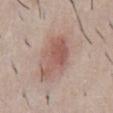Imaged during a routine full-body skin examination; the lesion was not biopsied and no histopathology is available.
Automated image analysis of the tile measured a footprint of about 13 mm². It also reported a border-irregularity index near 4/10 and internal color variation of about 4 on a 0–10 scale.
A 15 mm crop from a total-body photograph taken for skin-cancer surveillance.
Measured at roughly 5.5 mm in maximum diameter.
A male patient, about 50 years old.
The lesion is on the abdomen.